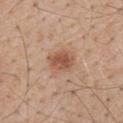follow-up: total-body-photography surveillance lesion; no biopsy | site: the mid back | acquisition: ~15 mm crop, total-body skin-cancer survey | patient: male, aged around 55.